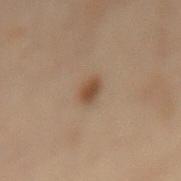{
  "biopsy_status": "not biopsied; imaged during a skin examination",
  "automated_metrics": {
    "area_mm2_approx": 3.5,
    "eccentricity": 0.8,
    "shape_asymmetry": 0.3,
    "cielab_L": 39,
    "cielab_a": 14,
    "cielab_b": 26,
    "vs_skin_darker_L": 9.0,
    "vs_skin_contrast_norm": 8.5,
    "nevus_likeness_0_100": 95,
    "lesion_detection_confidence_0_100": 100
  },
  "lesion_size": {
    "long_diameter_mm_approx": 2.5
  },
  "patient": {
    "sex": "female",
    "age_approx": 55
  },
  "site": "back",
  "image": {
    "source": "total-body photography crop",
    "field_of_view_mm": 15
  },
  "lighting": "cross-polarized"
}